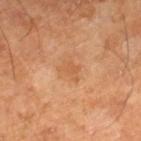notes: imaged on a skin check; not biopsied | image source: total-body-photography crop, ~15 mm field of view | anatomic site: the leg | lesion diameter: about 2.5 mm | patient: male, aged 58–62 | tile lighting: cross-polarized | TBP lesion metrics: a footprint of about 3.5 mm², an outline eccentricity of about 0.7 (0 = round, 1 = elongated), and two-axis asymmetry of about 0.45; border irregularity of about 4.5 on a 0–10 scale, internal color variation of about 0.5 on a 0–10 scale, and peripheral color asymmetry of about 0.